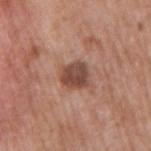No biopsy was performed on this lesion — it was imaged during a full skin examination and was not determined to be concerning. A 15 mm crop from a total-body photograph taken for skin-cancer surveillance. The lesion is on the right upper arm. A male patient approximately 70 years of age.